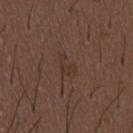Clinical impression: Captured during whole-body skin photography for melanoma surveillance; the lesion was not biopsied. Background: Cropped from a total-body skin-imaging series; the visible field is about 15 mm. The lesion is located on the abdomen. An algorithmic analysis of the crop reported a footprint of about 4 mm² and a shape-asymmetry score of about 0.55 (0 = symmetric). It also reported a lesion color around L≈33 a*≈16 b*≈22 in CIELAB. The analysis additionally found a border-irregularity index near 5.5/10, internal color variation of about 1.5 on a 0–10 scale, and a peripheral color-asymmetry measure near 0.5. The patient is a male roughly 50 years of age.